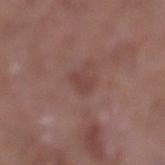Q: Is there a histopathology result?
A: catalogued during a skin exam; not biopsied
Q: What kind of image is this?
A: total-body-photography crop, ~15 mm field of view
Q: Illumination type?
A: white-light illumination
Q: Who is the patient?
A: male, aged 53 to 57
Q: Lesion location?
A: the left lower leg
Q: Lesion size?
A: ≈2.5 mm